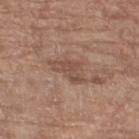The lesion was photographed on a routine skin check and not biopsied; there is no pathology result. The subject is a female in their mid- to late 70s. Captured under white-light illumination. Automated tile analysis of the lesion measured a classifier nevus-likeness of about 0/100 and a lesion-detection confidence of about 100/100. Approximately 4 mm at its widest. A 15 mm close-up tile from a total-body photography series done for melanoma screening. On the leg.